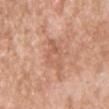{"biopsy_status": "not biopsied; imaged during a skin examination", "lighting": "white-light", "site": "chest", "lesion_size": {"long_diameter_mm_approx": 4.5}, "image": {"source": "total-body photography crop", "field_of_view_mm": 15}, "patient": {"sex": "male", "age_approx": 40}, "automated_metrics": {"area_mm2_approx": 6.0, "eccentricity": 0.9, "shape_asymmetry": 0.35, "cielab_L": 59, "cielab_a": 23, "cielab_b": 32, "vs_skin_darker_L": 7.0, "vs_skin_contrast_norm": 5.0, "border_irregularity_0_10": 5.0, "color_variation_0_10": 4.5, "peripheral_color_asymmetry": 1.5, "nevus_likeness_0_100": 0, "lesion_detection_confidence_0_100": 95}}